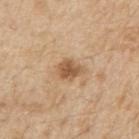Clinical summary: An algorithmic analysis of the crop reported a footprint of about 5 mm², an eccentricity of roughly 0.6, and a shape-asymmetry score of about 0.25 (0 = symmetric). It also reported border irregularity of about 2.5 on a 0–10 scale, a color-variation rating of about 2/10, and peripheral color asymmetry of about 0.5. And it measured a lesion-detection confidence of about 100/100. From the right upper arm. A region of skin cropped from a whole-body photographic capture, roughly 15 mm wide. Imaged with white-light lighting. About 3 mm across. The patient is a male aged 68–72.